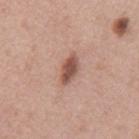Assessment: Imaged during a routine full-body skin examination; the lesion was not biopsied and no histopathology is available. Context: Approximately 3.5 mm at its widest. On the back. Cropped from a whole-body photographic skin survey; the tile spans about 15 mm. Captured under white-light illumination. A male subject approximately 40 years of age.